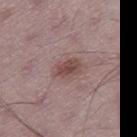{"biopsy_status": "not biopsied; imaged during a skin examination", "image": {"source": "total-body photography crop", "field_of_view_mm": 15}, "patient": {"sex": "male", "age_approx": 50}, "lighting": "white-light", "lesion_size": {"long_diameter_mm_approx": 3.5}, "site": "right thigh"}